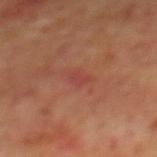Part of a total-body skin-imaging series; this lesion was reviewed on a skin check and was not flagged for biopsy. A male subject, roughly 70 years of age. Approximately 2.5 mm at its widest. This is a cross-polarized tile. On the mid back. A 15 mm close-up tile from a total-body photography series done for melanoma screening.